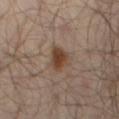workup: total-body-photography surveillance lesion; no biopsy | size: about 3 mm | image: 15 mm crop, total-body photography | subject: male, aged 63 to 67 | body site: the leg.